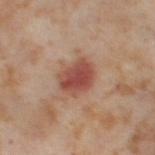Captured during whole-body skin photography for melanoma surveillance; the lesion was not biopsied. From the right thigh. The tile uses cross-polarized illumination. The subject is a female aged around 55. The lesion-visualizer software estimated a mean CIELAB color near L≈49 a*≈25 b*≈28, a lesion–skin lightness drop of about 12, and a lesion-to-skin contrast of about 8.5 (normalized; higher = more distinct). A 15 mm close-up extracted from a 3D total-body photography capture.